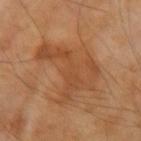notes — imaged on a skin check; not biopsied | site — the right forearm | automated metrics — a lesion area of about 27 mm², an eccentricity of roughly 0.6, and two-axis asymmetry of about 0.6; a border-irregularity index near 7/10 and a color-variation rating of about 3.5/10 | size — ≈8 mm | patient — male, aged around 70 | illumination — cross-polarized | imaging modality — ~15 mm crop, total-body skin-cancer survey.